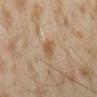Part of a total-body skin-imaging series; this lesion was reviewed on a skin check and was not flagged for biopsy. Imaged with cross-polarized lighting. Longest diameter approximately 2.5 mm. Cropped from a whole-body photographic skin survey; the tile spans about 15 mm. The patient is a male roughly 45 years of age. Located on the right forearm.